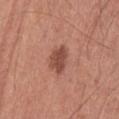| feature | finding |
|---|---|
| follow-up | total-body-photography surveillance lesion; no biopsy |
| subject | male, aged 63–67 |
| tile lighting | white-light |
| lesion diameter | ~3.5 mm (longest diameter) |
| image source | ~15 mm crop, total-body skin-cancer survey |
| automated lesion analysis | an area of roughly 6 mm², an eccentricity of roughly 0.7, and two-axis asymmetry of about 0.25; a lesion color around L≈48 a*≈25 b*≈28 in CIELAB and roughly 12 lightness units darker than nearby skin; a border-irregularity index near 2.5/10, a within-lesion color-variation index near 2.5/10, and radial color variation of about 1 |
| location | the left thigh |